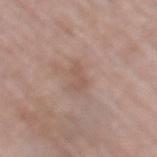Assessment:
This lesion was catalogued during total-body skin photography and was not selected for biopsy.
Image and clinical context:
On the upper back. A female patient, aged 58 to 62. A region of skin cropped from a whole-body photographic capture, roughly 15 mm wide.